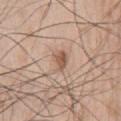Assessment: Imaged during a routine full-body skin examination; the lesion was not biopsied and no histopathology is available. Background: A male subject, aged 63–67. Imaged with white-light lighting. Approximately 3 mm at its widest. A close-up tile cropped from a whole-body skin photograph, about 15 mm across. Automated image analysis of the tile measured a lesion–skin lightness drop of about 11 and a lesion-to-skin contrast of about 7.5 (normalized; higher = more distinct). The software also gave a border-irregularity index near 3.5/10 and radial color variation of about 1. And it measured lesion-presence confidence of about 100/100. On the chest.